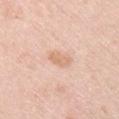{"biopsy_status": "not biopsied; imaged during a skin examination", "image": {"source": "total-body photography crop", "field_of_view_mm": 15}, "patient": {"sex": "female", "age_approx": 65}, "lighting": "white-light", "site": "right upper arm", "automated_metrics": {"nevus_likeness_0_100": 0, "lesion_detection_confidence_0_100": 100}}